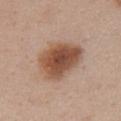– lighting — white-light illumination
– diameter — ~5.5 mm (longest diameter)
– site — the chest
– acquisition — ~15 mm tile from a whole-body skin photo
– subject — female, approximately 55 years of age